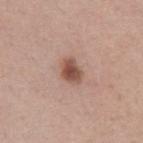notes = no biopsy performed (imaged during a skin exam)
subject = female, about 30 years old
anatomic site = the arm
diameter = ~3 mm (longest diameter)
automated metrics = a lesion area of about 5.5 mm² and a symmetry-axis asymmetry near 0.25; a classifier nevus-likeness of about 95/100 and a detector confidence of about 100 out of 100 that the crop contains a lesion
acquisition = ~15 mm crop, total-body skin-cancer survey